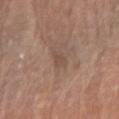Context:
From the right forearm. The patient is a female in their 60s. A lesion tile, about 15 mm wide, cut from a 3D total-body photograph.